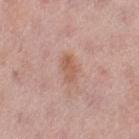notes = no biopsy performed (imaged during a skin exam); image source = ~15 mm crop, total-body skin-cancer survey; body site = the left thigh; patient = female, roughly 50 years of age; tile lighting = white-light illumination.